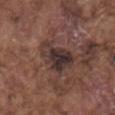The subject is a male aged approximately 75. Longest diameter approximately 5 mm. The tile uses white-light illumination. On the mid back. Cropped from a whole-body photographic skin survey; the tile spans about 15 mm.